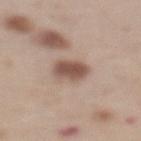{
  "biopsy_status": "not biopsied; imaged during a skin examination",
  "image": {
    "source": "total-body photography crop",
    "field_of_view_mm": 15
  },
  "automated_metrics": {
    "area_mm2_approx": 8.5,
    "eccentricity": 0.65,
    "cielab_L": 53,
    "cielab_a": 17,
    "cielab_b": 25,
    "vs_skin_darker_L": 13.0,
    "vs_skin_contrast_norm": 9.0,
    "nevus_likeness_0_100": 75
  },
  "patient": {
    "sex": "female",
    "age_approx": 65
  },
  "site": "back",
  "lighting": "white-light"
}